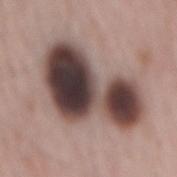Assessment:
This lesion was catalogued during total-body skin photography and was not selected for biopsy.
Context:
This is a white-light tile. The lesion's longest dimension is about 9.5 mm. From the back. The patient is a male aged 63–67. A 15 mm crop from a total-body photograph taken for skin-cancer surveillance.